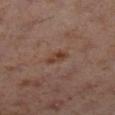Findings:
– biopsy status · catalogued during a skin exam; not biopsied
– patient · female, approximately 40 years of age
– site · the leg
– image source · ~15 mm crop, total-body skin-cancer survey
– lesion size · about 2.5 mm